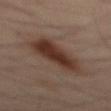Findings:
• notes: no biopsy performed (imaged during a skin exam)
• lighting: cross-polarized illumination
• image source: ~15 mm tile from a whole-body skin photo
• lesion size: ≈7.5 mm
• automated metrics: an automated nevus-likeness rating near 100 out of 100 and a detector confidence of about 100 out of 100 that the crop contains a lesion
• subject: male, aged approximately 50
• site: the mid back